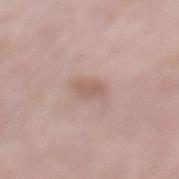Part of a total-body skin-imaging series; this lesion was reviewed on a skin check and was not flagged for biopsy. Captured under white-light illumination. A female patient, aged 68 to 72. A close-up tile cropped from a whole-body skin photograph, about 15 mm across. Located on the left lower leg. An algorithmic analysis of the crop reported a border-irregularity index near 3/10, a within-lesion color-variation index near 1.5/10, and peripheral color asymmetry of about 0.5. The software also gave a nevus-likeness score of about 25/100 and a lesion-detection confidence of about 100/100. About 3.5 mm across.